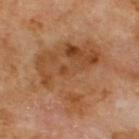The lesion was tiled from a total-body skin photograph and was not biopsied. The recorded lesion diameter is about 8.5 mm. The tile uses cross-polarized illumination. A 15 mm crop from a total-body photograph taken for skin-cancer surveillance. The subject is a male aged approximately 70. From the upper back. The lesion-visualizer software estimated a lesion area of about 37 mm², an eccentricity of roughly 0.55, and a shape-asymmetry score of about 0.55 (0 = symmetric). It also reported a lesion color around L≈47 a*≈21 b*≈35 in CIELAB, about 9 CIELAB-L* units darker than the surrounding skin, and a normalized border contrast of about 7.